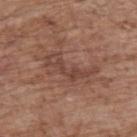Located on the upper back.
The recorded lesion diameter is about 6 mm.
The subject is a male aged 68 to 72.
Automated image analysis of the tile measured a classifier nevus-likeness of about 0/100 and a lesion-detection confidence of about 80/100.
A lesion tile, about 15 mm wide, cut from a 3D total-body photograph.